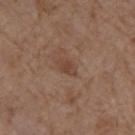The lesion was tiled from a total-body skin photograph and was not biopsied. Automated tile analysis of the lesion measured a nevus-likeness score of about 0/100 and lesion-presence confidence of about 100/100. Located on the left upper arm. A female subject aged 83 or older. Cropped from a whole-body photographic skin survey; the tile spans about 15 mm.